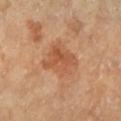<tbp_lesion>
<biopsy_status>not biopsied; imaged during a skin examination</biopsy_status>
<patient>
  <sex>female</sex>
  <age_approx>60</age_approx>
</patient>
<image>
  <source>total-body photography crop</source>
  <field_of_view_mm>15</field_of_view_mm>
</image>
<site>left lower leg</site>
<lighting>cross-polarized</lighting>
<lesion_size>
  <long_diameter_mm_approx>4.5</long_diameter_mm_approx>
</lesion_size>
</tbp_lesion>